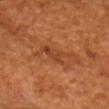| field | value |
|---|---|
| biopsy status | catalogued during a skin exam; not biopsied |
| patient | male, aged 58 to 62 |
| illumination | cross-polarized |
| image-analysis metrics | an eccentricity of roughly 0.9 and a shape-asymmetry score of about 0.45 (0 = symmetric); a classifier nevus-likeness of about 0/100 and a lesion-detection confidence of about 90/100 |
| size | ≈4.5 mm |
| body site | the front of the torso |
| image | 15 mm crop, total-body photography |